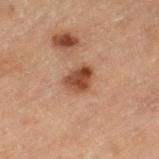biopsy status: total-body-photography surveillance lesion; no biopsy
location: the leg
diameter: ~3.5 mm (longest diameter)
subject: male, approximately 70 years of age
acquisition: total-body-photography crop, ~15 mm field of view
lighting: cross-polarized illumination